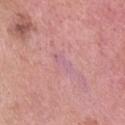Automated tile analysis of the lesion measured a footprint of about 3 mm², an outline eccentricity of about 0.95 (0 = round, 1 = elongated), and a shape-asymmetry score of about 0.45 (0 = symmetric). The software also gave a border-irregularity index near 5/10, a color-variation rating of about 0/10, and peripheral color asymmetry of about 0. The analysis additionally found a detector confidence of about 65 out of 100 that the crop contains a lesion.
A female subject aged 53 to 57.
Longest diameter approximately 3 mm.
Imaged with white-light lighting.
This image is a 15 mm lesion crop taken from a total-body photograph.
The lesion is located on the head or neck.